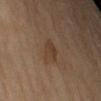Notes:
– workup — imaged on a skin check; not biopsied
– location — the left upper arm
– subject — female, in their 40s
– imaging modality — total-body-photography crop, ~15 mm field of view